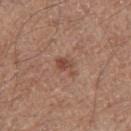Part of a total-body skin-imaging series; this lesion was reviewed on a skin check and was not flagged for biopsy.
Approximately 3 mm at its widest.
A 15 mm close-up tile from a total-body photography series done for melanoma screening.
A male subject approximately 65 years of age.
Imaged with white-light lighting.
The total-body-photography lesion software estimated an average lesion color of about L≈48 a*≈22 b*≈27 (CIELAB), a lesion–skin lightness drop of about 9, and a lesion-to-skin contrast of about 6.5 (normalized; higher = more distinct). And it measured a border-irregularity rating of about 4.5/10 and a within-lesion color-variation index near 3/10. The analysis additionally found a nevus-likeness score of about 50/100 and lesion-presence confidence of about 100/100.
Located on the left thigh.